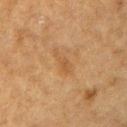workup = no biopsy performed (imaged during a skin exam) | lighting = cross-polarized illumination | site = the right upper arm | imaging modality = total-body-photography crop, ~15 mm field of view | lesion size = about 4 mm | subject = male, in their mid-70s.